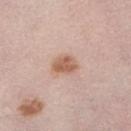The lesion was photographed on a routine skin check and not biopsied; there is no pathology result. The subject is a female aged 63–67. About 3 mm across. On the leg. A region of skin cropped from a whole-body photographic capture, roughly 15 mm wide. Automated tile analysis of the lesion measured an area of roughly 5.5 mm², an eccentricity of roughly 0.7, and a symmetry-axis asymmetry near 0.2. It also reported a nevus-likeness score of about 90/100 and lesion-presence confidence of about 100/100. The tile uses white-light illumination.